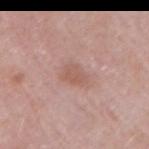  biopsy_status: not biopsied; imaged during a skin examination
  patient:
    sex: male
    age_approx: 55
  automated_metrics:
    area_mm2_approx: 5.0
    eccentricity: 0.65
    shape_asymmetry: 0.3
    cielab_L: 57
    cielab_a: 21
    cielab_b: 25
    border_irregularity_0_10: 3.0
    nevus_likeness_0_100: 5
    lesion_detection_confidence_0_100: 100
  lesion_size:
    long_diameter_mm_approx: 3.0
  lighting: white-light
  site: arm
  image:
    source: total-body photography crop
    field_of_view_mm: 15